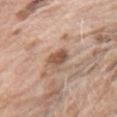{
  "biopsy_status": "not biopsied; imaged during a skin examination",
  "site": "arm",
  "patient": {
    "sex": "female",
    "age_approx": 75
  },
  "image": {
    "source": "total-body photography crop",
    "field_of_view_mm": 15
  },
  "lighting": "white-light",
  "automated_metrics": {
    "area_mm2_approx": 5.0,
    "eccentricity": 0.45,
    "shape_asymmetry": 0.25,
    "cielab_L": 53,
    "cielab_a": 19,
    "cielab_b": 29,
    "vs_skin_darker_L": 12.0,
    "vs_skin_contrast_norm": 8.5,
    "border_irregularity_0_10": 2.0,
    "color_variation_0_10": 3.0,
    "peripheral_color_asymmetry": 1.0
  },
  "lesion_size": {
    "long_diameter_mm_approx": 2.5
  }
}